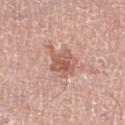{"biopsy_status": "not biopsied; imaged during a skin examination", "lesion_size": {"long_diameter_mm_approx": 4.0}, "site": "right lower leg", "lighting": "white-light", "patient": {"sex": "male", "age_approx": 75}, "image": {"source": "total-body photography crop", "field_of_view_mm": 15}}